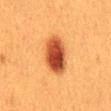Image and clinical context:
The patient is a female approximately 40 years of age. Approximately 5.5 mm at its widest. Cropped from a whole-body photographic skin survey; the tile spans about 15 mm. The tile uses cross-polarized illumination. The lesion is on the mid back.